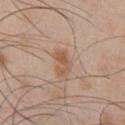Recorded during total-body skin imaging; not selected for excision or biopsy.
From the front of the torso.
A male subject aged 48–52.
This image is a 15 mm lesion crop taken from a total-body photograph.
Longest diameter approximately 3 mm.
An algorithmic analysis of the crop reported a lesion–skin lightness drop of about 8 and a normalized border contrast of about 7.